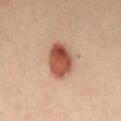Part of a total-body skin-imaging series; this lesion was reviewed on a skin check and was not flagged for biopsy.